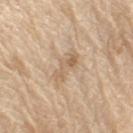biopsy_status: not biopsied; imaged during a skin examination
image:
  source: total-body photography crop
  field_of_view_mm: 15
lesion_size:
  long_diameter_mm_approx: 4.0
patient:
  sex: male
  age_approx: 70
site: right upper arm
automated_metrics:
  area_mm2_approx: 5.5
  eccentricity: 0.9
  shape_asymmetry: 0.4
  color_variation_0_10: 2.5
  peripheral_color_asymmetry: 0.5